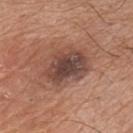- follow-up — imaged on a skin check; not biopsied
- patient — male, approximately 75 years of age
- automated lesion analysis — a lesion area of about 16 mm², an outline eccentricity of about 0.7 (0 = round, 1 = elongated), and a symmetry-axis asymmetry near 0.25; an average lesion color of about L≈44 a*≈19 b*≈24 (CIELAB), a lesion–skin lightness drop of about 12, and a lesion-to-skin contrast of about 10 (normalized; higher = more distinct); a border-irregularity rating of about 3/10 and peripheral color asymmetry of about 1.5
- image source — total-body-photography crop, ~15 mm field of view
- lighting — white-light
- body site — the chest